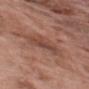Impression:
This lesion was catalogued during total-body skin photography and was not selected for biopsy.
Context:
Imaged with white-light lighting. A lesion tile, about 15 mm wide, cut from a 3D total-body photograph. The lesion is on the upper back. The patient is a male aged 68–72. The total-body-photography lesion software estimated a lesion color around L≈45 a*≈22 b*≈26 in CIELAB, about 8 CIELAB-L* units darker than the surrounding skin, and a lesion-to-skin contrast of about 6 (normalized; higher = more distinct).